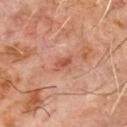Imaged with cross-polarized lighting. From the chest. A region of skin cropped from a whole-body photographic capture, roughly 15 mm wide. The patient is a male aged 58 to 62. Approximately 2.5 mm at its widest.